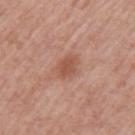The lesion was photographed on a routine skin check and not biopsied; there is no pathology result.
Approximately 3 mm at its widest.
A female patient approximately 60 years of age.
A roughly 15 mm field-of-view crop from a total-body skin photograph.
Located on the arm.
This is a white-light tile.